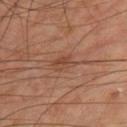Recorded during total-body skin imaging; not selected for excision or biopsy. About 2.5 mm across. The tile uses cross-polarized illumination. A male subject aged 63–67. A close-up tile cropped from a whole-body skin photograph, about 15 mm across. On the right thigh.